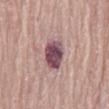{"biopsy_status": "not biopsied; imaged during a skin examination", "patient": {"sex": "female", "age_approx": 65}, "lesion_size": {"long_diameter_mm_approx": 4.0}, "lighting": "white-light", "site": "abdomen", "image": {"source": "total-body photography crop", "field_of_view_mm": 15}}